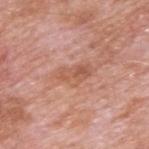Assessment: Imaged during a routine full-body skin examination; the lesion was not biopsied and no histopathology is available. Clinical summary: A 15 mm close-up tile from a total-body photography series done for melanoma screening. The lesion is located on the back. The recorded lesion diameter is about 4.5 mm. A male patient, aged 58–62.